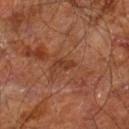Q: Is there a histopathology result?
A: catalogued during a skin exam; not biopsied
Q: What is the lesion's diameter?
A: ~2.5 mm (longest diameter)
Q: Automated lesion metrics?
A: a footprint of about 2.5 mm², an outline eccentricity of about 0.9 (0 = round, 1 = elongated), and a shape-asymmetry score of about 0.35 (0 = symmetric); an average lesion color of about L≈36 a*≈24 b*≈31 (CIELAB), a lesion–skin lightness drop of about 7, and a normalized border contrast of about 6.5; a lesion-detection confidence of about 100/100
Q: How was this image acquired?
A: ~15 mm tile from a whole-body skin photo
Q: Where on the body is the lesion?
A: the right leg
Q: Patient demographics?
A: male, aged around 60